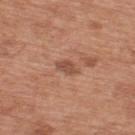This lesion was catalogued during total-body skin photography and was not selected for biopsy.
The lesion is located on the upper back.
A male subject, aged 68–72.
The recorded lesion diameter is about 3 mm.
A roughly 15 mm field-of-view crop from a total-body skin photograph.
Imaged with white-light lighting.